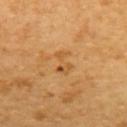Captured during whole-body skin photography for melanoma surveillance; the lesion was not biopsied. A lesion tile, about 15 mm wide, cut from a 3D total-body photograph. Captured under cross-polarized illumination. A female patient about 55 years old. The lesion is on the upper back.